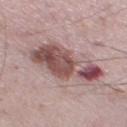Q: Was a biopsy performed?
A: imaged on a skin check; not biopsied
Q: Automated lesion metrics?
A: an area of roughly 22 mm², an eccentricity of roughly 0.9, and a shape-asymmetry score of about 0.35 (0 = symmetric); a lesion color around L≈50 a*≈21 b*≈19 in CIELAB, a lesion–skin lightness drop of about 15, and a normalized lesion–skin contrast near 10.5
Q: How was the tile lit?
A: white-light
Q: Lesion location?
A: the left thigh
Q: Lesion size?
A: ≈8 mm
Q: Patient demographics?
A: male, aged around 75
Q: How was this image acquired?
A: ~15 mm crop, total-body skin-cancer survey